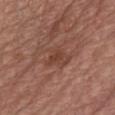Background:
An algorithmic analysis of the crop reported a footprint of about 6.5 mm², a shape eccentricity near 0.5, and a symmetry-axis asymmetry near 0.45. The analysis additionally found a lesion color around L≈42 a*≈23 b*≈27 in CIELAB. The software also gave a classifier nevus-likeness of about 0/100 and a lesion-detection confidence of about 100/100. The lesion is located on the chest. A close-up tile cropped from a whole-body skin photograph, about 15 mm across. Measured at roughly 3 mm in maximum diameter. A male subject, about 60 years old.